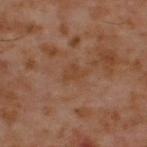Q: Was this lesion biopsied?
A: catalogued during a skin exam; not biopsied
Q: What are the patient's age and sex?
A: male, aged 58 to 62
Q: What did automated image analysis measure?
A: an area of roughly 3 mm² and an eccentricity of roughly 0.8; a lesion color around L≈39 a*≈20 b*≈30 in CIELAB; internal color variation of about 1 on a 0–10 scale and radial color variation of about 0.5; a nevus-likeness score of about 0/100 and a lesion-detection confidence of about 100/100
Q: What kind of image is this?
A: 15 mm crop, total-body photography
Q: What is the lesion's diameter?
A: ~2.5 mm (longest diameter)
Q: Where on the body is the lesion?
A: the upper back
Q: Illumination type?
A: cross-polarized